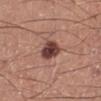follow-up: total-body-photography surveillance lesion; no biopsy | site: the left lower leg | patient: male, aged around 60 | automated lesion analysis: a lesion area of about 6.5 mm²; an average lesion color of about L≈41 a*≈22 b*≈23 (CIELAB), about 17 CIELAB-L* units darker than the surrounding skin, and a normalized border contrast of about 12.5; an automated nevus-likeness rating near 85 out of 100 and a lesion-detection confidence of about 100/100 | lesion size: ~3.5 mm (longest diameter) | imaging modality: total-body-photography crop, ~15 mm field of view.